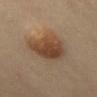body site: the back | lesion size: about 5 mm | patient: female, aged approximately 40 | illumination: cross-polarized illumination | imaging modality: total-body-photography crop, ~15 mm field of view | automated lesion analysis: a lesion color around L≈43 a*≈17 b*≈30 in CIELAB, roughly 10 lightness units darker than nearby skin, and a normalized lesion–skin contrast near 8.5; a border-irregularity index near 2.5/10, a color-variation rating of about 5/10, and radial color variation of about 1.5; a classifier nevus-likeness of about 100/100 and lesion-presence confidence of about 100/100.